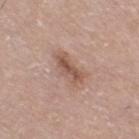biopsy_status: not biopsied; imaged during a skin examination
lesion_size:
  long_diameter_mm_approx: 4.0
patient:
  sex: male
  age_approx: 75
image:
  source: total-body photography crop
  field_of_view_mm: 15
lighting: white-light
site: right thigh